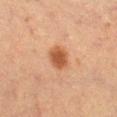Q: Was a biopsy performed?
A: imaged on a skin check; not biopsied
Q: Patient demographics?
A: female, aged 53–57
Q: How was this image acquired?
A: ~15 mm tile from a whole-body skin photo
Q: Where on the body is the lesion?
A: the left lower leg
Q: How large is the lesion?
A: about 3 mm
Q: Illumination type?
A: cross-polarized